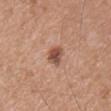This lesion was catalogued during total-body skin photography and was not selected for biopsy.
The lesion is located on the chest.
The subject is a male aged 38 to 42.
Captured under white-light illumination.
A 15 mm crop from a total-body photograph taken for skin-cancer surveillance.
The recorded lesion diameter is about 2.5 mm.